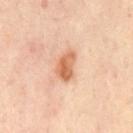biopsy status: catalogued during a skin exam; not biopsied
TBP lesion metrics: an area of roughly 6.5 mm², a shape eccentricity near 0.8, and a shape-asymmetry score of about 0.3 (0 = symmetric); a lesion–skin lightness drop of about 13 and a normalized border contrast of about 8.5; a nevus-likeness score of about 85/100
illumination: cross-polarized
body site: the chest
patient: roughly 55 years of age
size: about 3.5 mm
image: ~15 mm tile from a whole-body skin photo